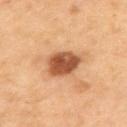{
  "biopsy_status": "not biopsied; imaged during a skin examination",
  "lighting": "cross-polarized",
  "patient": {
    "sex": "male",
    "age_approx": 55
  },
  "site": "upper back",
  "image": {
    "source": "total-body photography crop",
    "field_of_view_mm": 15
  },
  "lesion_size": {
    "long_diameter_mm_approx": 4.5
  },
  "automated_metrics": {
    "vs_skin_contrast_norm": 11.0,
    "nevus_likeness_0_100": 95,
    "lesion_detection_confidence_0_100": 100
  }
}